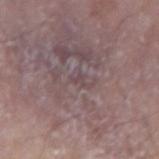Findings:
* workup · imaged on a skin check; not biopsied
* imaging modality · ~15 mm tile from a whole-body skin photo
* subject · male, in their mid- to late 60s
* image-analysis metrics · border irregularity of about 4.5 on a 0–10 scale, a within-lesion color-variation index near 0/10, and a peripheral color-asymmetry measure near 0
* site · the left forearm
* lighting · white-light
* lesion diameter · ≈1 mm